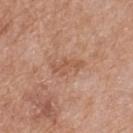No biopsy was performed on this lesion — it was imaged during a full skin examination and was not determined to be concerning. From the upper back. The tile uses white-light illumination. A female patient about 75 years old. Longest diameter approximately 4 mm. A 15 mm crop from a total-body photograph taken for skin-cancer surveillance.